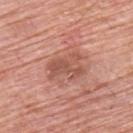Captured during whole-body skin photography for melanoma surveillance; the lesion was not biopsied.
Imaged with white-light lighting.
The lesion is on the upper back.
Cropped from a whole-body photographic skin survey; the tile spans about 15 mm.
A male subject, aged 58 to 62.